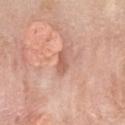anatomic site — the chest
patient — female, about 45 years old
TBP lesion metrics — a shape-asymmetry score of about 0.35 (0 = symmetric); a classifier nevus-likeness of about 0/100
image — total-body-photography crop, ~15 mm field of view